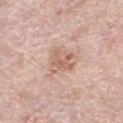Imaged during a routine full-body skin examination; the lesion was not biopsied and no histopathology is available. A female subject, approximately 65 years of age. Approximately 3.5 mm at its widest. A 15 mm close-up extracted from a 3D total-body photography capture. The tile uses white-light illumination. Located on the left lower leg.